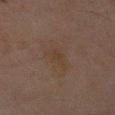Clinical impression: Recorded during total-body skin imaging; not selected for excision or biopsy. Acquisition and patient details: The subject is a male in their mid- to late 60s. The recorded lesion diameter is about 3 mm. Imaged with cross-polarized lighting. The lesion is located on the arm. Cropped from a whole-body photographic skin survey; the tile spans about 15 mm.